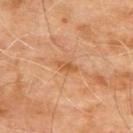biopsy_status: not biopsied; imaged during a skin examination
image:
  source: total-body photography crop
  field_of_view_mm: 15
lesion_size:
  long_diameter_mm_approx: 3.0
site: upper back
lighting: cross-polarized
patient:
  sex: male
  age_approx: 65